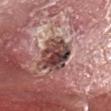imaging modality: ~15 mm tile from a whole-body skin photo; patient: male, aged approximately 65; TBP lesion metrics: a border-irregularity rating of about 2/10, a within-lesion color-variation index near 10/10, and a peripheral color-asymmetry measure near 3; lesion size: about 4 mm; site: the head or neck; lighting: white-light illumination; histopathologic diagnosis: an invasive squamous cell carcinoma, keratoacanthoma type.